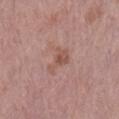Q: Lesion location?
A: the right lower leg
Q: What are the patient's age and sex?
A: female, aged 63 to 67
Q: How was this image acquired?
A: total-body-photography crop, ~15 mm field of view